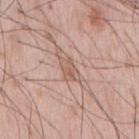Recorded during total-body skin imaging; not selected for excision or biopsy. The patient is a male about 65 years old. A 15 mm close-up tile from a total-body photography series done for melanoma screening. This is a white-light tile. The lesion is on the chest.